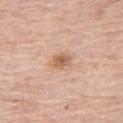Captured during whole-body skin photography for melanoma surveillance; the lesion was not biopsied.
A male subject aged approximately 80.
From the right thigh.
Automated tile analysis of the lesion measured an area of roughly 4.5 mm², an eccentricity of roughly 0.55, and a shape-asymmetry score of about 0.25 (0 = symmetric). It also reported a border-irregularity rating of about 2.5/10 and radial color variation of about 1.5. It also reported a nevus-likeness score of about 60/100.
A close-up tile cropped from a whole-body skin photograph, about 15 mm across.
Imaged with white-light lighting.